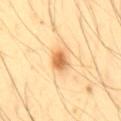Q: Is there a histopathology result?
A: no biopsy performed (imaged during a skin exam)
Q: Lesion size?
A: ≈3 mm
Q: Where on the body is the lesion?
A: the mid back
Q: How was this image acquired?
A: 15 mm crop, total-body photography
Q: Who is the patient?
A: male, aged around 45